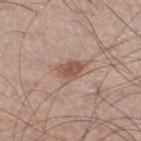biopsy_status: not biopsied; imaged during a skin examination
lighting: white-light
patient:
  sex: male
  age_approx: 60
automated_metrics:
  nevus_likeness_0_100: 55
  lesion_detection_confidence_0_100: 100
site: right thigh
lesion_size:
  long_diameter_mm_approx: 3.0
image:
  source: total-body photography crop
  field_of_view_mm: 15